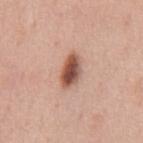Captured during whole-body skin photography for melanoma surveillance; the lesion was not biopsied. Imaged with white-light lighting. Automated image analysis of the tile measured a shape eccentricity near 0.85 and a symmetry-axis asymmetry near 0.2. Located on the chest. Cropped from a whole-body photographic skin survey; the tile spans about 15 mm. A male patient, approximately 60 years of age. Measured at roughly 4 mm in maximum diameter.